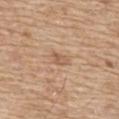Q: What is the anatomic site?
A: the front of the torso
Q: What is the imaging modality?
A: ~15 mm crop, total-body skin-cancer survey
Q: Who is the patient?
A: male, about 70 years old